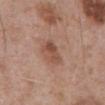Assessment: Imaged during a routine full-body skin examination; the lesion was not biopsied and no histopathology is available. Background: Located on the front of the torso. The patient is a male aged approximately 70. A roughly 15 mm field-of-view crop from a total-body skin photograph. Longest diameter approximately 3.5 mm. Imaged with white-light lighting.